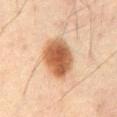Recorded during total-body skin imaging; not selected for excision or biopsy.
A male patient, aged 63 to 67.
From the mid back.
A region of skin cropped from a whole-body photographic capture, roughly 15 mm wide.
Captured under cross-polarized illumination.
The lesion's longest dimension is about 5.5 mm.